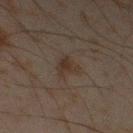Q: Was this lesion biopsied?
A: catalogued during a skin exam; not biopsied